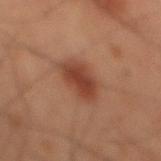| feature | finding |
|---|---|
| notes | no biopsy performed (imaged during a skin exam) |
| imaging modality | total-body-photography crop, ~15 mm field of view |
| patient | male, aged 58 to 62 |
| location | the back |